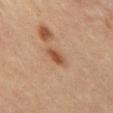| field | value |
|---|---|
| notes | catalogued during a skin exam; not biopsied |
| imaging modality | 15 mm crop, total-body photography |
| lighting | cross-polarized |
| location | the right upper arm |
| subject | female, about 45 years old |
| TBP lesion metrics | an eccentricity of roughly 0.8 and a symmetry-axis asymmetry near 0.2; border irregularity of about 2 on a 0–10 scale, a within-lesion color-variation index near 2.5/10, and a peripheral color-asymmetry measure near 0.5; a nevus-likeness score of about 90/100 and lesion-presence confidence of about 100/100 |
| lesion diameter | ≈3 mm |